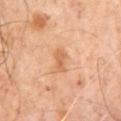Assessment:
This lesion was catalogued during total-body skin photography and was not selected for biopsy.
Clinical summary:
A male subject, in their mid- to late 60s. About 3 mm across. A 15 mm crop from a total-body photograph taken for skin-cancer surveillance. The tile uses cross-polarized illumination.